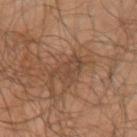Imaged during a routine full-body skin examination; the lesion was not biopsied and no histopathology is available.
A lesion tile, about 15 mm wide, cut from a 3D total-body photograph.
The patient is a male about 65 years old.
From the left upper arm.